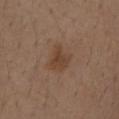| feature | finding |
|---|---|
| anatomic site | the chest |
| imaging modality | ~15 mm crop, total-body skin-cancer survey |
| patient | male, about 75 years old |